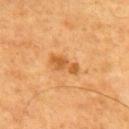lighting = cross-polarized illumination
subject = male, approximately 60 years of age
automated lesion analysis = a footprint of about 5 mm² and two-axis asymmetry of about 0.4; a within-lesion color-variation index near 1.5/10
lesion diameter = ~3.5 mm (longest diameter)
anatomic site = the mid back
image source = ~15 mm tile from a whole-body skin photo